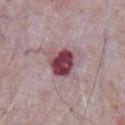notes: total-body-photography surveillance lesion; no biopsy | image: 15 mm crop, total-body photography | patient: male, aged 63 to 67 | tile lighting: white-light | site: the chest | image-analysis metrics: an average lesion color of about L≈43 a*≈27 b*≈14 (CIELAB), about 19 CIELAB-L* units darker than the surrounding skin, and a lesion-to-skin contrast of about 13.5 (normalized; higher = more distinct); a border-irregularity index near 2/10, a color-variation rating of about 6/10, and a peripheral color-asymmetry measure near 2; an automated nevus-likeness rating near 0 out of 100 and a detector confidence of about 100 out of 100 that the crop contains a lesion.